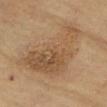A roughly 15 mm field-of-view crop from a total-body skin photograph. The lesion is located on the arm. The patient is a male in their mid- to late 60s. About 8 mm across.